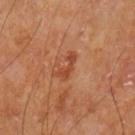Clinical impression: No biopsy was performed on this lesion — it was imaged during a full skin examination and was not determined to be concerning. Clinical summary: An algorithmic analysis of the crop reported a lesion color around L≈44 a*≈27 b*≈35 in CIELAB and about 8 CIELAB-L* units darker than the surrounding skin. And it measured a nevus-likeness score of about 0/100 and lesion-presence confidence of about 100/100. The patient is a male aged 63–67. Approximately 3 mm at its widest. The lesion is located on the left upper arm. Imaged with cross-polarized lighting. Cropped from a whole-body photographic skin survey; the tile spans about 15 mm.